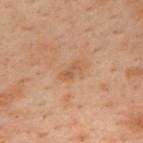Findings:
– workup: catalogued during a skin exam; not biopsied
– body site: the upper back
– lesion size: ~2.5 mm (longest diameter)
– subject: female, roughly 55 years of age
– illumination: cross-polarized illumination
– acquisition: 15 mm crop, total-body photography
– image-analysis metrics: an average lesion color of about L≈47 a*≈18 b*≈31 (CIELAB) and roughly 6 lightness units darker than nearby skin; a detector confidence of about 100 out of 100 that the crop contains a lesion